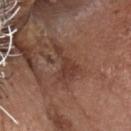notes = no biopsy performed (imaged during a skin exam)
tile lighting = white-light
body site = the head or neck
acquisition = 15 mm crop, total-body photography
subject = male, in their mid-70s
TBP lesion metrics = a lesion area of about 6.5 mm², a shape eccentricity near 0.85, and two-axis asymmetry of about 0.7; border irregularity of about 8.5 on a 0–10 scale, a color-variation rating of about 1.5/10, and a peripheral color-asymmetry measure near 0.5; an automated nevus-likeness rating near 0 out of 100
lesion size = about 4.5 mm